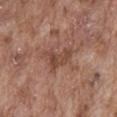Clinical impression:
No biopsy was performed on this lesion — it was imaged during a full skin examination and was not determined to be concerning.
Context:
The tile uses white-light illumination. The lesion is located on the abdomen. A lesion tile, about 15 mm wide, cut from a 3D total-body photograph. A male patient aged 73–77. The lesion's longest dimension is about 4 mm.